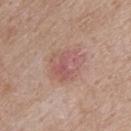<tbp_lesion>
<biopsy_status>not biopsied; imaged during a skin examination</biopsy_status>
<image>
  <source>total-body photography crop</source>
  <field_of_view_mm>15</field_of_view_mm>
</image>
<site>upper back</site>
<patient>
  <sex>male</sex>
  <age_approx>75</age_approx>
</patient>
</tbp_lesion>